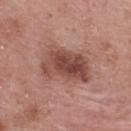follow-up = total-body-photography surveillance lesion; no biopsy
imaging modality = ~15 mm crop, total-body skin-cancer survey
lighting = white-light
size = ≈6 mm
anatomic site = the upper back
patient = male, aged approximately 65